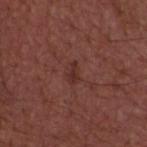Q: Was a biopsy performed?
A: total-body-photography surveillance lesion; no biopsy
Q: What are the patient's age and sex?
A: male, aged approximately 50
Q: Where on the body is the lesion?
A: the upper back
Q: What kind of image is this?
A: ~15 mm crop, total-body skin-cancer survey
Q: Illumination type?
A: white-light